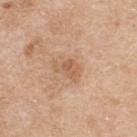This lesion was catalogued during total-body skin photography and was not selected for biopsy.
The tile uses white-light illumination.
The lesion's longest dimension is about 3 mm.
Cropped from a total-body skin-imaging series; the visible field is about 15 mm.
From the upper back.
The total-body-photography lesion software estimated a mean CIELAB color near L≈59 a*≈20 b*≈34 and a lesion-to-skin contrast of about 5.5 (normalized; higher = more distinct). The software also gave lesion-presence confidence of about 100/100.
A male subject, aged approximately 60.